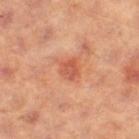biopsy status=no biopsy performed (imaged during a skin exam) | subject=female, aged 38–42 | anatomic site=the right thigh | image=~15 mm tile from a whole-body skin photo.